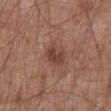notes — no biopsy performed (imaged during a skin exam); body site — the mid back; illumination — white-light illumination; subject — male, roughly 60 years of age; diameter — about 3 mm; image — ~15 mm tile from a whole-body skin photo.